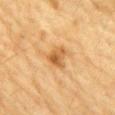Part of a total-body skin-imaging series; this lesion was reviewed on a skin check and was not flagged for biopsy. A male patient, aged 83 or older. The lesion is located on the front of the torso. A roughly 15 mm field-of-view crop from a total-body skin photograph. Imaged with cross-polarized lighting. An algorithmic analysis of the crop reported a lesion color around L≈51 a*≈20 b*≈40 in CIELAB, roughly 10 lightness units darker than nearby skin, and a lesion-to-skin contrast of about 7.5 (normalized; higher = more distinct). And it measured border irregularity of about 3.5 on a 0–10 scale, a color-variation rating of about 5.5/10, and radial color variation of about 2. It also reported a classifier nevus-likeness of about 35/100 and lesion-presence confidence of about 100/100.